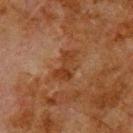Part of a total-body skin-imaging series; this lesion was reviewed on a skin check and was not flagged for biopsy. The lesion is located on the upper back. The recorded lesion diameter is about 4 mm. The lesion-visualizer software estimated a footprint of about 7.5 mm² and an eccentricity of roughly 0.85. And it measured a lesion color around L≈30 a*≈20 b*≈29 in CIELAB, a lesion–skin lightness drop of about 6, and a lesion-to-skin contrast of about 7 (normalized; higher = more distinct). And it measured a nevus-likeness score of about 0/100 and a detector confidence of about 100 out of 100 that the crop contains a lesion. A 15 mm crop from a total-body photograph taken for skin-cancer surveillance. The patient is a male approximately 80 years of age.